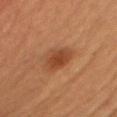Clinical impression:
The lesion was tiled from a total-body skin photograph and was not biopsied.
Image and clinical context:
A 15 mm close-up tile from a total-body photography series done for melanoma screening. From the front of the torso. A male patient, approximately 50 years of age.